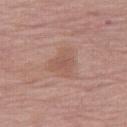{
  "biopsy_status": "not biopsied; imaged during a skin examination",
  "patient": {
    "sex": "female",
    "age_approx": 65
  },
  "site": "right thigh",
  "automated_metrics": {
    "area_mm2_approx": 9.0,
    "shape_asymmetry": 0.45,
    "cielab_L": 55,
    "cielab_a": 20,
    "cielab_b": 26,
    "vs_skin_darker_L": 6.0,
    "vs_skin_contrast_norm": 5.0,
    "border_irregularity_0_10": 4.0,
    "color_variation_0_10": 2.5,
    "peripheral_color_asymmetry": 0.5
  },
  "image": {
    "source": "total-body photography crop",
    "field_of_view_mm": 15
  },
  "lighting": "white-light",
  "lesion_size": {
    "long_diameter_mm_approx": 4.0
  }
}